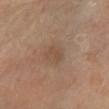A close-up tile cropped from a whole-body skin photograph, about 15 mm across. A female patient aged around 65. From the right leg.